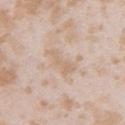Clinical impression:
Recorded during total-body skin imaging; not selected for excision or biopsy.
Context:
A 15 mm close-up extracted from a 3D total-body photography capture. A female patient, aged 23 to 27. Located on the left upper arm.